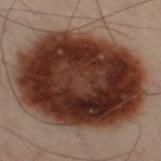<lesion>
<biopsy_status>not biopsied; imaged during a skin examination</biopsy_status>
<lighting>cross-polarized</lighting>
<automated_metrics>
  <border_irregularity_0_10>1.0</border_irregularity_0_10>
  <peripheral_color_asymmetry>2.0</peripheral_color_asymmetry>
  <nevus_likeness_0_100>95</nevus_likeness_0_100>
  <lesion_detection_confidence_0_100>95</lesion_detection_confidence_0_100>
</automated_metrics>
<site>leg</site>
<lesion_size>
  <long_diameter_mm_approx>12.5</long_diameter_mm_approx>
</lesion_size>
<image>
  <source>total-body photography crop</source>
  <field_of_view_mm>15</field_of_view_mm>
</image>
<patient>
  <sex>male</sex>
  <age_approx>50</age_approx>
</patient>
</lesion>